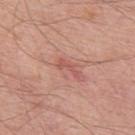notes — imaged on a skin check; not biopsied | patient — male, approximately 60 years of age | illumination — white-light | TBP lesion metrics — a mean CIELAB color near L≈55 a*≈28 b*≈27, roughly 8 lightness units darker than nearby skin, and a normalized lesion–skin contrast near 5.5; internal color variation of about 0 on a 0–10 scale and peripheral color asymmetry of about 0; a lesion-detection confidence of about 100/100 | acquisition — total-body-photography crop, ~15 mm field of view | size — ~2.5 mm (longest diameter) | site — the upper back.